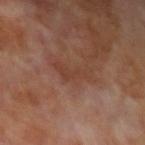This lesion was catalogued during total-body skin photography and was not selected for biopsy. Located on the left upper arm. Automated tile analysis of the lesion measured a lesion area of about 5 mm², an eccentricity of roughly 0.95, and a shape-asymmetry score of about 0.55 (0 = symmetric). The software also gave an average lesion color of about L≈39 a*≈21 b*≈27 (CIELAB), about 5 CIELAB-L* units darker than the surrounding skin, and a normalized border contrast of about 5. The software also gave a border-irregularity rating of about 6.5/10, internal color variation of about 0.5 on a 0–10 scale, and radial color variation of about 0. A 15 mm crop from a total-body photograph taken for skin-cancer surveillance. The patient is a male about 70 years old.